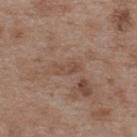The lesion was tiled from a total-body skin photograph and was not biopsied. The lesion is on the upper back. A 15 mm crop from a total-body photograph taken for skin-cancer surveillance. A male patient, aged around 50.